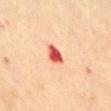Q: What are the patient's age and sex?
A: female, in their 60s
Q: Lesion size?
A: ≈3 mm
Q: Where on the body is the lesion?
A: the abdomen
Q: What kind of image is this?
A: 15 mm crop, total-body photography